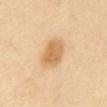workup: total-body-photography surveillance lesion; no biopsy | subject: female, roughly 60 years of age | body site: the abdomen | image source: ~15 mm crop, total-body skin-cancer survey.